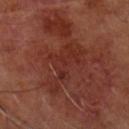follow-up: catalogued during a skin exam; not biopsied | illumination: cross-polarized | lesion diameter: about 7 mm | subject: male, in their 70s | anatomic site: the arm | acquisition: 15 mm crop, total-body photography.